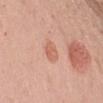* lighting — white-light
* patient — female, aged approximately 40
* image — 15 mm crop, total-body photography
* site — the left upper arm
* lesion diameter — ≈2.5 mm